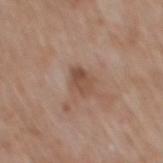Assessment:
Imaged during a routine full-body skin examination; the lesion was not biopsied and no histopathology is available.
Background:
Measured at roughly 3 mm in maximum diameter. The subject is a male roughly 60 years of age. The lesion is on the mid back. A lesion tile, about 15 mm wide, cut from a 3D total-body photograph. This is a white-light tile. The total-body-photography lesion software estimated a normalized border contrast of about 7. And it measured a classifier nevus-likeness of about 0/100 and lesion-presence confidence of about 100/100.